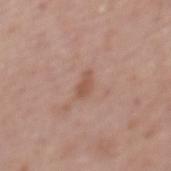A 15 mm crop from a total-body photograph taken for skin-cancer surveillance. The lesion's longest dimension is about 2.5 mm. Captured under white-light illumination. A male patient aged around 70. On the mid back.